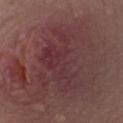No biopsy was performed on this lesion — it was imaged during a full skin examination and was not determined to be concerning. Longest diameter approximately 8 mm. Automated tile analysis of the lesion measured about 5 CIELAB-L* units darker than the surrounding skin and a normalized lesion–skin contrast near 5.5. The analysis additionally found a border-irregularity index near 8/10, internal color variation of about 5 on a 0–10 scale, and peripheral color asymmetry of about 2. The subject is a female about 40 years old. The tile uses white-light illumination. A 15 mm crop from a total-body photograph taken for skin-cancer surveillance. The lesion is located on the front of the torso.